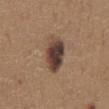notes: total-body-photography surveillance lesion; no biopsy | image: total-body-photography crop, ~15 mm field of view | site: the chest | patient: male, approximately 65 years of age.